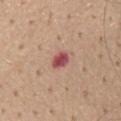{
  "biopsy_status": "not biopsied; imaged during a skin examination"
}